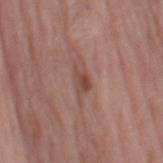Clinical impression:
Imaged during a routine full-body skin examination; the lesion was not biopsied and no histopathology is available.
Context:
On the right thigh. A close-up tile cropped from a whole-body skin photograph, about 15 mm across. The subject is a female aged around 70.